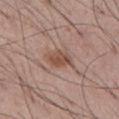Recorded during total-body skin imaging; not selected for excision or biopsy.
From the abdomen.
This is a white-light tile.
A region of skin cropped from a whole-body photographic capture, roughly 15 mm wide.
The patient is a male in their mid- to late 50s.
The lesion's longest dimension is about 3 mm.